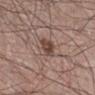<tbp_lesion>
  <biopsy_status>not biopsied; imaged during a skin examination</biopsy_status>
  <patient>
    <sex>male</sex>
    <age_approx>50</age_approx>
  </patient>
  <site>right lower leg</site>
  <image>
    <source>total-body photography crop</source>
    <field_of_view_mm>15</field_of_view_mm>
  </image>
  <lesion_size>
    <long_diameter_mm_approx>3.0</long_diameter_mm_approx>
  </lesion_size>
  <lighting>white-light</lighting>
  <automated_metrics>
    <area_mm2_approx>5.5</area_mm2_approx>
    <eccentricity>0.7</eccentricity>
    <shape_asymmetry>0.25</shape_asymmetry>
    <cielab_L>45</cielab_L>
    <cielab_a>18</cielab_a>
    <cielab_b>23</cielab_b>
    <vs_skin_darker_L>11.0</vs_skin_darker_L>
    <vs_skin_contrast_norm>8.5</vs_skin_contrast_norm>
    <nevus_likeness_0_100>95</nevus_likeness_0_100>
    <lesion_detection_confidence_0_100>100</lesion_detection_confidence_0_100>
  </automated_metrics>
</tbp_lesion>